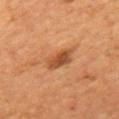A male patient, roughly 55 years of age. The total-body-photography lesion software estimated a footprint of about 6.5 mm² and an outline eccentricity of about 0.8 (0 = round, 1 = elongated). And it measured about 11 CIELAB-L* units darker than the surrounding skin and a lesion-to-skin contrast of about 8 (normalized; higher = more distinct). It also reported a border-irregularity rating of about 2.5/10, internal color variation of about 4.5 on a 0–10 scale, and a peripheral color-asymmetry measure near 1.5. On the mid back. Captured under cross-polarized illumination. A 15 mm close-up tile from a total-body photography series done for melanoma screening.